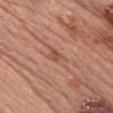illumination: white-light; acquisition: ~15 mm tile from a whole-body skin photo; patient: female, aged 58–62; size: ~2.5 mm (longest diameter); anatomic site: the chest.